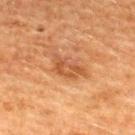- biopsy status · no biopsy performed (imaged during a skin exam)
- image-analysis metrics · a footprint of about 7.5 mm², an eccentricity of roughly 0.85, and a symmetry-axis asymmetry near 0.35; a lesion color around L≈44 a*≈22 b*≈34 in CIELAB and a lesion-to-skin contrast of about 6 (normalized; higher = more distinct); a border-irregularity index near 4/10 and radial color variation of about 1
- tile lighting · cross-polarized
- subject · male, aged around 50
- anatomic site · the upper back
- size · ~4.5 mm (longest diameter)
- acquisition · total-body-photography crop, ~15 mm field of view